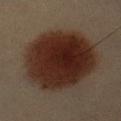The patient is a male roughly 40 years of age. Automated image analysis of the tile measured an area of roughly 55 mm², a shape eccentricity near 0.6, and a symmetry-axis asymmetry near 0.1. The analysis additionally found a border-irregularity index near 1.5/10 and a color-variation rating of about 5/10. The analysis additionally found a nevus-likeness score of about 100/100 and a lesion-detection confidence of about 100/100. A close-up tile cropped from a whole-body skin photograph, about 15 mm across. Imaged with cross-polarized lighting. From the left upper arm. The lesion's longest dimension is about 9.5 mm.